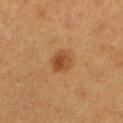Impression:
This lesion was catalogued during total-body skin photography and was not selected for biopsy.
Background:
Captured under cross-polarized illumination. The lesion is located on the left lower leg. A region of skin cropped from a whole-body photographic capture, roughly 15 mm wide. The subject is a female aged around 40. Longest diameter approximately 2.5 mm. Automated image analysis of the tile measured an area of roughly 5.5 mm², an eccentricity of roughly 0.45, and two-axis asymmetry of about 0.15. The analysis additionally found a nevus-likeness score of about 95/100 and a lesion-detection confidence of about 100/100.